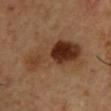{"biopsy_status": "not biopsied; imaged during a skin examination", "patient": {"sex": "male", "age_approx": 55}, "lesion_size": {"long_diameter_mm_approx": 8.5}, "site": "right upper arm", "image": {"source": "total-body photography crop", "field_of_view_mm": 15}, "automated_metrics": {"area_mm2_approx": 26.0, "eccentricity": 0.9, "shape_asymmetry": 0.35, "cielab_L": 35, "cielab_a": 19, "cielab_b": 29, "vs_skin_darker_L": 11.0, "vs_skin_contrast_norm": 9.5, "border_irregularity_0_10": 4.5, "color_variation_0_10": 10.0, "nevus_likeness_0_100": 85, "lesion_detection_confidence_0_100": 100}}